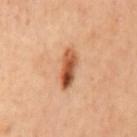Recorded during total-body skin imaging; not selected for excision or biopsy. A roughly 15 mm field-of-view crop from a total-body skin photograph. From the mid back.